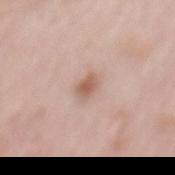This lesion was catalogued during total-body skin photography and was not selected for biopsy.
The subject is a female aged 63–67.
This is a white-light tile.
Longest diameter approximately 2.5 mm.
Located on the mid back.
A close-up tile cropped from a whole-body skin photograph, about 15 mm across.